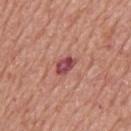Findings:
– automated metrics: internal color variation of about 5.5 on a 0–10 scale and a peripheral color-asymmetry measure near 2
– imaging modality: 15 mm crop, total-body photography
– lesion size: ≈3 mm
– illumination: white-light
– subject: male, approximately 70 years of age
– body site: the upper back